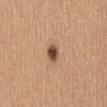This lesion was catalogued during total-body skin photography and was not selected for biopsy.
A close-up tile cropped from a whole-body skin photograph, about 15 mm across.
This is a white-light tile.
Located on the lower back.
A female subject in their 60s.
Automated image analysis of the tile measured an area of roughly 4 mm² and a shape-asymmetry score of about 0.1 (0 = symmetric). The analysis additionally found an average lesion color of about L≈49 a*≈20 b*≈29 (CIELAB), a lesion–skin lightness drop of about 16, and a lesion-to-skin contrast of about 11.5 (normalized; higher = more distinct).
About 2.5 mm across.